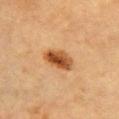Imaged during a routine full-body skin examination; the lesion was not biopsied and no histopathology is available. Measured at roughly 4 mm in maximum diameter. A male patient, about 85 years old or older. This is a cross-polarized tile. The lesion is located on the chest. Cropped from a total-body skin-imaging series; the visible field is about 15 mm.